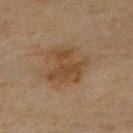Impression:
Recorded during total-body skin imaging; not selected for excision or biopsy.
Acquisition and patient details:
A lesion tile, about 15 mm wide, cut from a 3D total-body photograph. On the upper back. The tile uses cross-polarized illumination. A female patient, aged approximately 70.